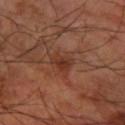Impression:
This lesion was catalogued during total-body skin photography and was not selected for biopsy.
Context:
Captured under cross-polarized illumination. A male patient about 65 years old. Longest diameter approximately 3 mm. A close-up tile cropped from a whole-body skin photograph, about 15 mm across. The lesion is located on the arm. Automated tile analysis of the lesion measured a mean CIELAB color near L≈34 a*≈23 b*≈29 and a lesion-to-skin contrast of about 7 (normalized; higher = more distinct). And it measured border irregularity of about 3 on a 0–10 scale, a within-lesion color-variation index near 3/10, and radial color variation of about 1.